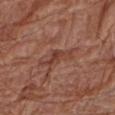follow-up: no biopsy performed (imaged during a skin exam) | patient: female, aged 78 to 82 | imaging modality: ~15 mm crop, total-body skin-cancer survey | lesion diameter: ~4 mm (longest diameter) | location: the right forearm | lighting: white-light illumination.